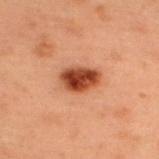{"biopsy_status": "not biopsied; imaged during a skin examination", "image": {"source": "total-body photography crop", "field_of_view_mm": 15}, "patient": {"sex": "male", "age_approx": 55}, "site": "upper back"}